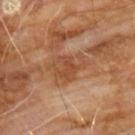Clinical impression:
No biopsy was performed on this lesion — it was imaged during a full skin examination and was not determined to be concerning.
Background:
Measured at roughly 5 mm in maximum diameter. An algorithmic analysis of the crop reported a lesion area of about 12 mm². The analysis additionally found a border-irregularity rating of about 5/10, a color-variation rating of about 4/10, and radial color variation of about 1. The subject is a male about 60 years old. From the chest. Cropped from a total-body skin-imaging series; the visible field is about 15 mm. Imaged with cross-polarized lighting.